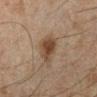Part of a total-body skin-imaging series; this lesion was reviewed on a skin check and was not flagged for biopsy.
This image is a 15 mm lesion crop taken from a total-body photograph.
A male subject, in their mid-40s.
On the left lower leg.
An algorithmic analysis of the crop reported an average lesion color of about L≈35 a*≈14 b*≈24 (CIELAB), a lesion–skin lightness drop of about 9, and a lesion-to-skin contrast of about 8.5 (normalized; higher = more distinct). The software also gave a border-irregularity index near 2.5/10 and a within-lesion color-variation index near 3/10. And it measured a nevus-likeness score of about 90/100 and lesion-presence confidence of about 100/100.
Longest diameter approximately 3.5 mm.